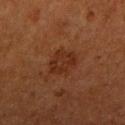Impression: This lesion was catalogued during total-body skin photography and was not selected for biopsy. Background: The lesion is located on the right upper arm. The tile uses cross-polarized illumination. About 3 mm across. The subject is a female aged approximately 50. A 15 mm close-up tile from a total-body photography series done for melanoma screening. The lesion-visualizer software estimated an automated nevus-likeness rating near 10 out of 100 and a detector confidence of about 100 out of 100 that the crop contains a lesion.